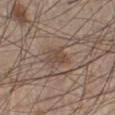Q: Was this lesion biopsied?
A: total-body-photography surveillance lesion; no biopsy
Q: How was this image acquired?
A: 15 mm crop, total-body photography
Q: What lighting was used for the tile?
A: white-light illumination
Q: What are the patient's age and sex?
A: male, aged 58 to 62
Q: How large is the lesion?
A: about 3 mm
Q: Where on the body is the lesion?
A: the left lower leg